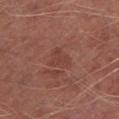Notes:
– biopsy status — imaged on a skin check; not biopsied
– lighting — white-light illumination
– image — 15 mm crop, total-body photography
– patient — male, aged around 65
– location — the left lower leg